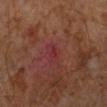Clinical impression: Captured during whole-body skin photography for melanoma surveillance; the lesion was not biopsied. Background: A male patient aged around 60. Imaged with cross-polarized lighting. Cropped from a total-body skin-imaging series; the visible field is about 15 mm. The lesion is located on the left lower leg. Longest diameter approximately 2.5 mm.